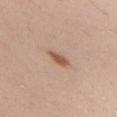{
  "biopsy_status": "not biopsied; imaged during a skin examination",
  "patient": {
    "sex": "male",
    "age_approx": 70
  },
  "site": "upper back",
  "image": {
    "source": "total-body photography crop",
    "field_of_view_mm": 15
  },
  "lesion_size": {
    "long_diameter_mm_approx": 3.0
  },
  "lighting": "white-light"
}